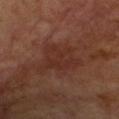workup: total-body-photography surveillance lesion; no biopsy
subject: female, aged 58 to 62
lesion diameter: ~6 mm (longest diameter)
image: total-body-photography crop, ~15 mm field of view
body site: the chest